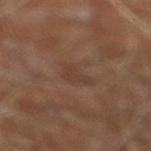Case summary:
– biopsy status — total-body-photography surveillance lesion; no biopsy
– diameter — ~3 mm (longest diameter)
– site — the right leg
– automated metrics — a shape eccentricity near 0.75 and a shape-asymmetry score of about 0.6 (0 = symmetric); border irregularity of about 6 on a 0–10 scale and peripheral color asymmetry of about 0.5
– acquisition — ~15 mm crop, total-body skin-cancer survey
– patient — male, approximately 60 years of age